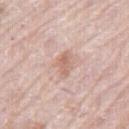Notes:
– notes — no biopsy performed (imaged during a skin exam)
– image — total-body-photography crop, ~15 mm field of view
– patient — male, aged approximately 80
– lighting — white-light illumination
– site — the right thigh
– lesion diameter — ~3.5 mm (longest diameter)
– automated lesion analysis — a shape eccentricity near 0.75 and a symmetry-axis asymmetry near 0.3; a within-lesion color-variation index near 3/10 and radial color variation of about 1; lesion-presence confidence of about 100/100